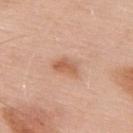Background: A 15 mm close-up extracted from a 3D total-body photography capture. Located on the back. The subject is a male aged 53–57. The tile uses white-light illumination. Measured at roughly 3 mm in maximum diameter.